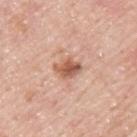Q: How large is the lesion?
A: ≈3 mm
Q: Where on the body is the lesion?
A: the upper back
Q: Who is the patient?
A: male, approximately 45 years of age
Q: How was this image acquired?
A: 15 mm crop, total-body photography
Q: How was the tile lit?
A: white-light